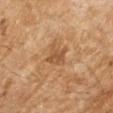Q: Was this lesion biopsied?
A: no biopsy performed (imaged during a skin exam)
Q: How large is the lesion?
A: ≈3 mm
Q: Lesion location?
A: the right forearm
Q: Patient demographics?
A: female, in their 70s
Q: How was this image acquired?
A: ~15 mm crop, total-body skin-cancer survey